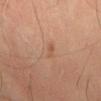<lesion>
  <biopsy_status>not biopsied; imaged during a skin examination</biopsy_status>
  <lighting>cross-polarized</lighting>
  <lesion_size>
    <long_diameter_mm_approx>3.0</long_diameter_mm_approx>
  </lesion_size>
  <automated_metrics>
    <border_irregularity_0_10>4.5</border_irregularity_0_10>
    <color_variation_0_10>0.0</color_variation_0_10>
    <lesion_detection_confidence_0_100>100</lesion_detection_confidence_0_100>
  </automated_metrics>
  <image>
    <source>total-body photography crop</source>
    <field_of_view_mm>15</field_of_view_mm>
  </image>
  <patient>
    <sex>male</sex>
    <age_approx>65</age_approx>
  </patient>
</lesion>